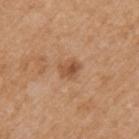Notes:
• follow-up: imaged on a skin check; not biopsied
• image source: ~15 mm tile from a whole-body skin photo
• subject: male, roughly 70 years of age
• location: the arm